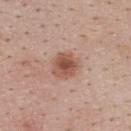Imaged during a routine full-body skin examination; the lesion was not biopsied and no histopathology is available. A female patient, roughly 25 years of age. This is a white-light tile. Approximately 3.5 mm at its widest. The lesion is located on the upper back. A lesion tile, about 15 mm wide, cut from a 3D total-body photograph.